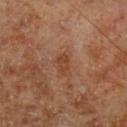Findings:
• notes · no biopsy performed (imaged during a skin exam)
• image source · ~15 mm tile from a whole-body skin photo
• patient · male, about 70 years old
• lesion size · ≈2.5 mm
• TBP lesion metrics · a lesion area of about 3.5 mm², a shape eccentricity near 0.85, and two-axis asymmetry of about 0.25; an average lesion color of about L≈41 a*≈23 b*≈32 (CIELAB), a lesion–skin lightness drop of about 7, and a lesion-to-skin contrast of about 6.5 (normalized; higher = more distinct); a border-irregularity index near 2.5/10, a color-variation rating of about 1.5/10, and radial color variation of about 0.5
• site · the leg